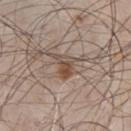Part of a total-body skin-imaging series; this lesion was reviewed on a skin check and was not flagged for biopsy. The recorded lesion diameter is about 3.5 mm. The tile uses white-light illumination. A lesion tile, about 15 mm wide, cut from a 3D total-body photograph. A male patient aged approximately 55. On the chest.